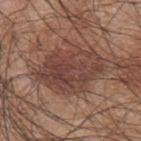Impression: Part of a total-body skin-imaging series; this lesion was reviewed on a skin check and was not flagged for biopsy. Context: This is a white-light tile. The total-body-photography lesion software estimated a lesion area of about 24 mm², a shape eccentricity near 0.8, and a symmetry-axis asymmetry near 0.25. The software also gave a border-irregularity index near 4/10 and a peripheral color-asymmetry measure near 2. A male patient, in their mid- to late 40s. Cropped from a total-body skin-imaging series; the visible field is about 15 mm. The lesion is located on the upper back. Longest diameter approximately 7.5 mm.